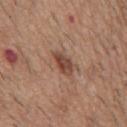Case summary:
• notes: no biopsy performed (imaged during a skin exam)
• anatomic site: the mid back
• image source: ~15 mm crop, total-body skin-cancer survey
• illumination: white-light
• subject: male, aged around 60